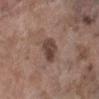Recorded during total-body skin imaging; not selected for excision or biopsy.